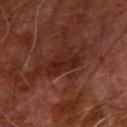Assessment: Part of a total-body skin-imaging series; this lesion was reviewed on a skin check and was not flagged for biopsy. Acquisition and patient details: The tile uses cross-polarized illumination. A roughly 15 mm field-of-view crop from a total-body skin photograph. A male subject approximately 80 years of age. Located on the left forearm.